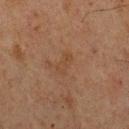Automated tile analysis of the lesion measured a lesion area of about 4 mm² and a shape eccentricity near 0.85. The analysis additionally found a border-irregularity rating of about 3/10, a within-lesion color-variation index near 2/10, and peripheral color asymmetry of about 0.5. Imaged with cross-polarized lighting. On the chest. The subject is a male about 75 years old. A close-up tile cropped from a whole-body skin photograph, about 15 mm across. Measured at roughly 3 mm in maximum diameter.